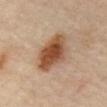Clinical impression:
The lesion was tiled from a total-body skin photograph and was not biopsied.
Acquisition and patient details:
Automated tile analysis of the lesion measured a footprint of about 15 mm², a shape eccentricity near 0.8, and a symmetry-axis asymmetry near 0.2. The software also gave roughly 15 lightness units darker than nearby skin and a normalized lesion–skin contrast near 11. The analysis additionally found border irregularity of about 2.5 on a 0–10 scale and internal color variation of about 6 on a 0–10 scale. The software also gave a detector confidence of about 100 out of 100 that the crop contains a lesion. Approximately 5.5 mm at its widest. This is a cross-polarized tile. A male subject aged 63 to 67. The lesion is located on the abdomen. A 15 mm close-up tile from a total-body photography series done for melanoma screening.